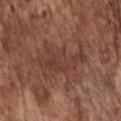follow-up: catalogued during a skin exam; not biopsied
image-analysis metrics: a lesion area of about 12 mm², a shape eccentricity near 0.9, and a symmetry-axis asymmetry near 0.4; a border-irregularity rating of about 7.5/10, a color-variation rating of about 2.5/10, and radial color variation of about 0.5; a classifier nevus-likeness of about 0/100
size: ~6 mm (longest diameter)
imaging modality: total-body-photography crop, ~15 mm field of view
tile lighting: white-light illumination
body site: the chest
patient: male, approximately 75 years of age